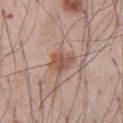No biopsy was performed on this lesion — it was imaged during a full skin examination and was not determined to be concerning.
The lesion's longest dimension is about 3.5 mm.
A lesion tile, about 15 mm wide, cut from a 3D total-body photograph.
This is a white-light tile.
Located on the abdomen.
A male subject about 55 years old.
The total-body-photography lesion software estimated a lesion color around L≈53 a*≈20 b*≈27 in CIELAB. And it measured border irregularity of about 2.5 on a 0–10 scale and a peripheral color-asymmetry measure near 1.